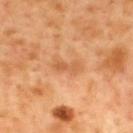Impression:
The lesion was tiled from a total-body skin photograph and was not biopsied.
Acquisition and patient details:
A male patient, aged 48 to 52. The lesion is on the upper back. Cropped from a total-body skin-imaging series; the visible field is about 15 mm.